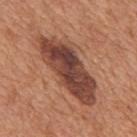| field | value |
|---|---|
| notes | no biopsy performed (imaged during a skin exam) |
| tile lighting | white-light illumination |
| body site | the mid back |
| subject | male, in their mid-60s |
| lesion diameter | about 9.5 mm |
| acquisition | total-body-photography crop, ~15 mm field of view |